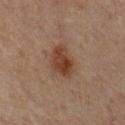Q: Was a biopsy performed?
A: total-body-photography surveillance lesion; no biopsy
Q: What is the imaging modality?
A: ~15 mm crop, total-body skin-cancer survey
Q: What did automated image analysis measure?
A: a lesion color around L≈31 a*≈16 b*≈24 in CIELAB, a lesion–skin lightness drop of about 8, and a normalized lesion–skin contrast near 8.5; a classifier nevus-likeness of about 90/100 and a detector confidence of about 100 out of 100 that the crop contains a lesion
Q: Where on the body is the lesion?
A: the mid back
Q: Who is the patient?
A: male, in their mid-60s
Q: What is the lesion's diameter?
A: ~4.5 mm (longest diameter)